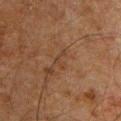Clinical impression: Recorded during total-body skin imaging; not selected for excision or biopsy. Context: Cropped from a whole-body photographic skin survey; the tile spans about 15 mm. On the chest. A male subject, aged 58 to 62. This is a cross-polarized tile.